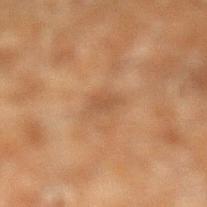* patient: male, about 60 years old
* location: the left lower leg
* lighting: cross-polarized illumination
* imaging modality: ~15 mm tile from a whole-body skin photo